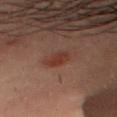notes = imaged on a skin check; not biopsied | image = total-body-photography crop, ~15 mm field of view | patient = male, aged 28 to 32 | site = the head or neck | tile lighting = cross-polarized illumination.